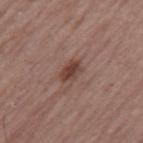{
  "image": {
    "source": "total-body photography crop",
    "field_of_view_mm": 15
  },
  "patient": {
    "sex": "male",
    "age_approx": 65
  },
  "site": "right thigh"
}